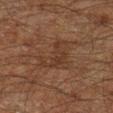follow-up = catalogued during a skin exam; not biopsied.